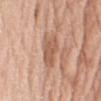The lesion was photographed on a routine skin check and not biopsied; there is no pathology result. The tile uses white-light illumination. About 4 mm across. Cropped from a total-body skin-imaging series; the visible field is about 15 mm. Located on the right upper arm. A male patient, aged 58 to 62.